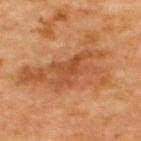Q: Was this lesion biopsied?
A: imaged on a skin check; not biopsied
Q: What is the imaging modality?
A: total-body-photography crop, ~15 mm field of view
Q: Who is the patient?
A: about 65 years old
Q: Lesion location?
A: the upper back